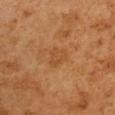• biopsy status — no biopsy performed (imaged during a skin exam)
• anatomic site — the right upper arm
• patient — male, aged around 50
• image — 15 mm crop, total-body photography
• tile lighting — cross-polarized
• lesion diameter — ~2.5 mm (longest diameter)